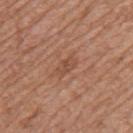Clinical impression:
Recorded during total-body skin imaging; not selected for excision or biopsy.
Context:
Approximately 3 mm at its widest. Imaged with white-light lighting. The subject is a female in their mid- to late 70s. From the left upper arm. A 15 mm crop from a total-body photograph taken for skin-cancer surveillance. Automated tile analysis of the lesion measured an area of roughly 4 mm², an eccentricity of roughly 0.8, and two-axis asymmetry of about 0.25. And it measured a classifier nevus-likeness of about 0/100 and a detector confidence of about 100 out of 100 that the crop contains a lesion.